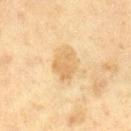Findings:
* notes — total-body-photography surveillance lesion; no biopsy
* patient — female, in their 50s
* illumination — cross-polarized
* diameter — ~3.5 mm (longest diameter)
* image source — 15 mm crop, total-body photography
* TBP lesion metrics — a footprint of about 7 mm², a shape eccentricity near 0.5, and a shape-asymmetry score of about 0.3 (0 = symmetric); an average lesion color of about L≈60 a*≈14 b*≈37 (CIELAB) and roughly 8 lightness units darker than nearby skin; a nevus-likeness score of about 5/100
* body site — the right lower leg